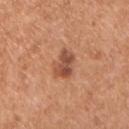Case summary:
* biopsy status: total-body-photography surveillance lesion; no biopsy
* acquisition: 15 mm crop, total-body photography
* patient: male, approximately 55 years of age
* body site: the chest
* lesion diameter: ≈3.5 mm